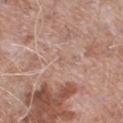Case summary:
• workup: no biopsy performed (imaged during a skin exam)
• lesion diameter: ~1 mm (longest diameter)
• anatomic site: the left lower leg
• image source: 15 mm crop, total-body photography
• subject: male, approximately 75 years of age
• tile lighting: white-light illumination